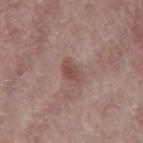The lesion was tiled from a total-body skin photograph and was not biopsied. The recorded lesion diameter is about 3 mm. Located on the back. A region of skin cropped from a whole-body photographic capture, roughly 15 mm wide. The patient is a male aged 68–72.